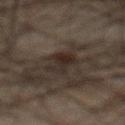Q: Was this lesion biopsied?
A: catalogued during a skin exam; not biopsied
Q: How large is the lesion?
A: ≈4 mm
Q: Lesion location?
A: the abdomen
Q: What is the imaging modality?
A: total-body-photography crop, ~15 mm field of view
Q: Who is the patient?
A: male, in their mid- to late 60s